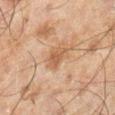biopsy status: catalogued during a skin exam; not biopsied | body site: the right lower leg | patient: male, in their mid- to late 40s | size: ≈2.5 mm | tile lighting: cross-polarized | image-analysis metrics: a mean CIELAB color near L≈46 a*≈18 b*≈29; a border-irregularity index near 3/10 and radial color variation of about 0.5; an automated nevus-likeness rating near 0 out of 100 and a lesion-detection confidence of about 100/100 | image source: 15 mm crop, total-body photography.